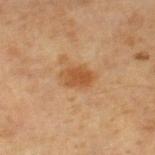workup = total-body-photography surveillance lesion; no biopsy
location = the right lower leg
subject = male, aged 58–62
automated metrics = a lesion color around L≈49 a*≈22 b*≈37 in CIELAB and a normalized border contrast of about 7.5; a classifier nevus-likeness of about 75/100 and a lesion-detection confidence of about 100/100
imaging modality = 15 mm crop, total-body photography
size = about 4 mm
illumination = cross-polarized illumination